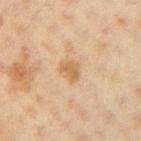The lesion was tiled from a total-body skin photograph and was not biopsied. A 15 mm close-up tile from a total-body photography series done for melanoma screening. A female patient aged approximately 20. The lesion is on the right upper arm.